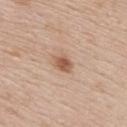follow-up: no biopsy performed (imaged during a skin exam) | subject: female, approximately 50 years of age | body site: the back | image source: ~15 mm tile from a whole-body skin photo.